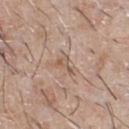The lesion was photographed on a routine skin check and not biopsied; there is no pathology result. Located on the chest. A 15 mm close-up tile from a total-body photography series done for melanoma screening. A male subject, roughly 65 years of age.